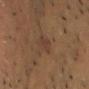Clinical impression:
The lesion was tiled from a total-body skin photograph and was not biopsied.
Clinical summary:
On the chest. The lesion-visualizer software estimated a border-irregularity index near 3.5/10. A male patient aged around 45. A roughly 15 mm field-of-view crop from a total-body skin photograph. Imaged with cross-polarized lighting.